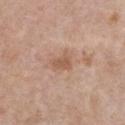The lesion was tiled from a total-body skin photograph and was not biopsied.
The lesion-visualizer software estimated an area of roughly 4.5 mm². And it measured a border-irregularity index near 3/10, a within-lesion color-variation index near 1.5/10, and radial color variation of about 0.5.
This is a white-light tile.
The lesion's longest dimension is about 3 mm.
The patient is a female aged around 65.
A close-up tile cropped from a whole-body skin photograph, about 15 mm across.
On the chest.